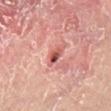Acquisition and patient details:
A roughly 15 mm field-of-view crop from a total-body skin photograph. A male patient roughly 55 years of age. From the leg. About 3 mm across. An algorithmic analysis of the crop reported a shape eccentricity near 0.8 and two-axis asymmetry of about 0.25. The analysis additionally found a lesion–skin lightness drop of about 11 and a normalized border contrast of about 8. This is a cross-polarized tile.